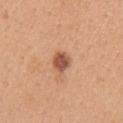{
  "site": "arm",
  "lighting": "white-light",
  "lesion_size": {
    "long_diameter_mm_approx": 2.5
  },
  "image": {
    "source": "total-body photography crop",
    "field_of_view_mm": 15
  },
  "automated_metrics": {
    "nevus_likeness_0_100": 85
  },
  "patient": {
    "sex": "female",
    "age_approx": 30
  }
}